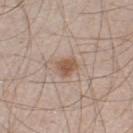Q: Where on the body is the lesion?
A: the left lower leg
Q: What is the imaging modality?
A: ~15 mm tile from a whole-body skin photo
Q: Illumination type?
A: white-light illumination
Q: Patient demographics?
A: male, about 60 years old